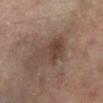lighting: cross-polarized | imaging modality: ~15 mm tile from a whole-body skin photo | location: the right lower leg | subject: female, aged approximately 75 | diameter: ≈5.5 mm.